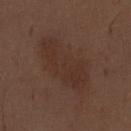Imaged during a routine full-body skin examination; the lesion was not biopsied and no histopathology is available. The lesion's longest dimension is about 8.5 mm. The tile uses white-light illumination. The subject is a male aged 68–72. From the abdomen. Automated image analysis of the tile measured a lesion area of about 28 mm², an eccentricity of roughly 0.85, and a symmetry-axis asymmetry near 0.25. It also reported an average lesion color of about L≈32 a*≈17 b*≈23 (CIELAB), roughly 5 lightness units darker than nearby skin, and a normalized border contrast of about 5. The analysis additionally found border irregularity of about 3.5 on a 0–10 scale, a color-variation rating of about 2/10, and radial color variation of about 1. Cropped from a total-body skin-imaging series; the visible field is about 15 mm.